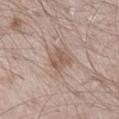workup: catalogued during a skin exam; not biopsied
subject: male, about 60 years old
site: the right lower leg
image source: ~15 mm crop, total-body skin-cancer survey